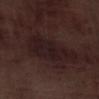Q: Was this lesion biopsied?
A: total-body-photography surveillance lesion; no biopsy
Q: Lesion location?
A: the left lower leg
Q: How was this image acquired?
A: total-body-photography crop, ~15 mm field of view
Q: Patient demographics?
A: male, aged 68 to 72
Q: How large is the lesion?
A: ≈7 mm
Q: Automated lesion metrics?
A: an area of roughly 18 mm² and a symmetry-axis asymmetry near 0.3; internal color variation of about 2.5 on a 0–10 scale